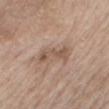This lesion was catalogued during total-body skin photography and was not selected for biopsy. A female patient aged around 75. On the mid back. A close-up tile cropped from a whole-body skin photograph, about 15 mm across.